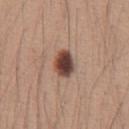Impression: Captured during whole-body skin photography for melanoma surveillance; the lesion was not biopsied. Image and clinical context: Approximately 3.5 mm at its widest. A male subject roughly 25 years of age. On the front of the torso. A 15 mm close-up tile from a total-body photography series done for melanoma screening. The lesion-visualizer software estimated a footprint of about 6.5 mm² and an eccentricity of roughly 0.65.